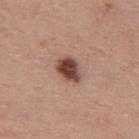image source = ~15 mm tile from a whole-body skin photo | patient = male, about 40 years old | diameter = ~3.5 mm (longest diameter) | anatomic site = the upper back | lighting = white-light.